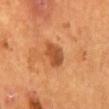No biopsy was performed on this lesion — it was imaged during a full skin examination and was not determined to be concerning. A 15 mm close-up extracted from a 3D total-body photography capture. Imaged with cross-polarized lighting. Located on the mid back. A male patient aged around 55. Approximately 3.5 mm at its widest.